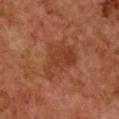follow-up: imaged on a skin check; not biopsied
subject: female, roughly 60 years of age
site: the chest
acquisition: total-body-photography crop, ~15 mm field of view